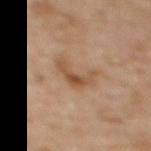Clinical impression:
This lesion was catalogued during total-body skin photography and was not selected for biopsy.
Context:
A female subject, roughly 60 years of age. On the upper back. An algorithmic analysis of the crop reported a border-irregularity rating of about 7/10, a color-variation rating of about 4/10, and peripheral color asymmetry of about 1. The analysis additionally found a nevus-likeness score of about 0/100 and a detector confidence of about 100 out of 100 that the crop contains a lesion. A close-up tile cropped from a whole-body skin photograph, about 15 mm across. This is a cross-polarized tile. Approximately 4 mm at its widest.